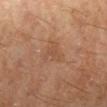Recorded during total-body skin imaging; not selected for excision or biopsy.
A male patient aged 58–62.
Captured under cross-polarized illumination.
From the right lower leg.
A close-up tile cropped from a whole-body skin photograph, about 15 mm across.